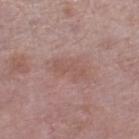Part of a total-body skin-imaging series; this lesion was reviewed on a skin check and was not flagged for biopsy. A region of skin cropped from a whole-body photographic capture, roughly 15 mm wide. A female subject, roughly 60 years of age. On the right lower leg.